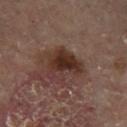notes: no biopsy performed (imaged during a skin exam) | automated metrics: a lesion area of about 9.5 mm², an outline eccentricity of about 0.7 (0 = round, 1 = elongated), and two-axis asymmetry of about 0.45; an average lesion color of about L≈30 a*≈18 b*≈22 (CIELAB) and a lesion-to-skin contrast of about 10.5 (normalized; higher = more distinct); a detector confidence of about 100 out of 100 that the crop contains a lesion | patient: female, aged 58 to 62 | location: the left lower leg | imaging modality: total-body-photography crop, ~15 mm field of view | size: ~4.5 mm (longest diameter).